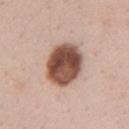Findings:
– illumination — white-light illumination
– lesion size — ~5.5 mm (longest diameter)
– subject — female, aged around 40
– anatomic site — the left upper arm
– acquisition — ~15 mm tile from a whole-body skin photo